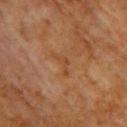workup = total-body-photography surveillance lesion; no biopsy | size = ~3.5 mm (longest diameter) | illumination = cross-polarized illumination | subject = female, aged approximately 80 | site = the upper back | image source = ~15 mm crop, total-body skin-cancer survey | TBP lesion metrics = a lesion-detection confidence of about 100/100.